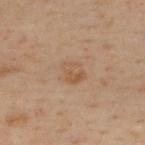notes = catalogued during a skin exam; not biopsied
patient = male, in their 50s
image source = ~15 mm crop, total-body skin-cancer survey
automated lesion analysis = a border-irregularity rating of about 2/10 and radial color variation of about 1; an automated nevus-likeness rating near 0 out of 100
tile lighting = cross-polarized
anatomic site = the upper back